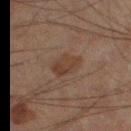biopsy status: total-body-photography surveillance lesion; no biopsy
anatomic site: the left leg
image-analysis metrics: an area of roughly 6.5 mm², an outline eccentricity of about 0.7 (0 = round, 1 = elongated), and a symmetry-axis asymmetry near 0.2; a mean CIELAB color near L≈28 a*≈13 b*≈21, a lesion–skin lightness drop of about 6, and a normalized border contrast of about 6.5
size: ~3.5 mm (longest diameter)
lighting: cross-polarized
subject: male, aged around 60
imaging modality: 15 mm crop, total-body photography